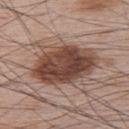Assessment: The lesion was photographed on a routine skin check and not biopsied; there is no pathology result. Acquisition and patient details: A 15 mm crop from a total-body photograph taken for skin-cancer surveillance. On the upper back. A male patient in their mid- to late 60s. Automated image analysis of the tile measured an eccentricity of roughly 0.7 and a shape-asymmetry score of about 0.2 (0 = symmetric). The software also gave an average lesion color of about L≈45 a*≈19 b*≈25 (CIELAB). The analysis additionally found a within-lesion color-variation index near 7/10 and peripheral color asymmetry of about 2.5.